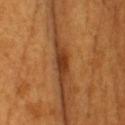image = 15 mm crop, total-body photography
subject = male, roughly 60 years of age
lighting = cross-polarized illumination
body site = the head or neck
diameter = ~4 mm (longest diameter)